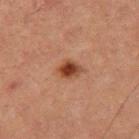<case>
<biopsy_status>not biopsied; imaged during a skin examination</biopsy_status>
<automated_metrics>
  <cielab_L>35</cielab_L>
  <cielab_a>21</cielab_a>
  <cielab_b>28</cielab_b>
  <vs_skin_darker_L>11.0</vs_skin_darker_L>
  <vs_skin_contrast_norm>10.0</vs_skin_contrast_norm>
  <border_irregularity_0_10>1.5</border_irregularity_0_10>
  <color_variation_0_10>5.0</color_variation_0_10>
  <peripheral_color_asymmetry>1.5</peripheral_color_asymmetry>
  <nevus_likeness_0_100>95</nevus_likeness_0_100>
  <lesion_detection_confidence_0_100>100</lesion_detection_confidence_0_100>
</automated_metrics>
<lighting>cross-polarized</lighting>
<patient>
  <sex>female</sex>
  <age_approx>60</age_approx>
</patient>
<site>right thigh</site>
<lesion_size>
  <long_diameter_mm_approx>3.0</long_diameter_mm_approx>
</lesion_size>
<image>
  <source>total-body photography crop</source>
  <field_of_view_mm>15</field_of_view_mm>
</image>
</case>